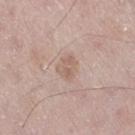No biopsy was performed on this lesion — it was imaged during a full skin examination and was not determined to be concerning.
A male patient, aged approximately 65.
The recorded lesion diameter is about 2.5 mm.
The lesion is on the right thigh.
A 15 mm crop from a total-body photograph taken for skin-cancer surveillance.
Imaged with white-light lighting.